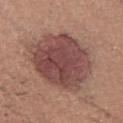Q: Was this lesion biopsied?
A: no biopsy performed (imaged during a skin exam)
Q: Patient demographics?
A: female, aged around 35
Q: Automated lesion metrics?
A: border irregularity of about 2 on a 0–10 scale and a peripheral color-asymmetry measure near 1.5
Q: What is the anatomic site?
A: the chest
Q: What lighting was used for the tile?
A: white-light illumination
Q: How was this image acquired?
A: ~15 mm crop, total-body skin-cancer survey